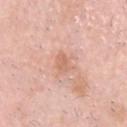Part of a total-body skin-imaging series; this lesion was reviewed on a skin check and was not flagged for biopsy.
Measured at roughly 2.5 mm in maximum diameter.
A 15 mm crop from a total-body photograph taken for skin-cancer surveillance.
A male patient about 70 years old.
Imaged with white-light lighting.
The total-body-photography lesion software estimated a lesion area of about 3.5 mm², a shape eccentricity near 0.65, and a shape-asymmetry score of about 0.3 (0 = symmetric). The software also gave a mean CIELAB color near L≈65 a*≈24 b*≈31, about 8 CIELAB-L* units darker than the surrounding skin, and a lesion-to-skin contrast of about 5.5 (normalized; higher = more distinct). And it measured border irregularity of about 2.5 on a 0–10 scale.
The lesion is on the head or neck.